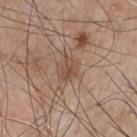Clinical impression: Part of a total-body skin-imaging series; this lesion was reviewed on a skin check and was not flagged for biopsy.